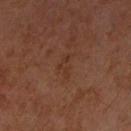Impression:
Part of a total-body skin-imaging series; this lesion was reviewed on a skin check and was not flagged for biopsy.
Image and clinical context:
Automated tile analysis of the lesion measured a footprint of about 4 mm² and an outline eccentricity of about 0.85 (0 = round, 1 = elongated). The software also gave internal color variation of about 1 on a 0–10 scale and radial color variation of about 0.5. Located on the right upper arm. A 15 mm crop from a total-body photograph taken for skin-cancer surveillance. Imaged with cross-polarized lighting. The patient is a female roughly 60 years of age. The recorded lesion diameter is about 3 mm.